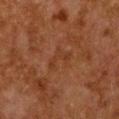Assessment:
Part of a total-body skin-imaging series; this lesion was reviewed on a skin check and was not flagged for biopsy.
Image and clinical context:
A roughly 15 mm field-of-view crop from a total-body skin photograph. The lesion is located on the chest. This is a cross-polarized tile. A female patient aged approximately 50. Longest diameter approximately 3.5 mm.